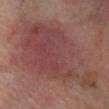Background: On the right lower leg. Cropped from a total-body skin-imaging series; the visible field is about 15 mm. Measured at roughly 14.5 mm in maximum diameter. This is a cross-polarized tile. A male patient, in their 70s.